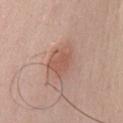Captured during whole-body skin photography for melanoma surveillance; the lesion was not biopsied.
On the front of the torso.
Imaged with white-light lighting.
The recorded lesion diameter is about 4.5 mm.
Cropped from a whole-body photographic skin survey; the tile spans about 15 mm.
The subject is a male in their 60s.